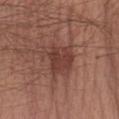| feature | finding |
|---|---|
| biopsy status | total-body-photography surveillance lesion; no biopsy |
| image | ~15 mm tile from a whole-body skin photo |
| patient | male, aged approximately 20 |
| site | the left forearm |